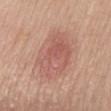Notes:
– workup — imaged on a skin check; not biopsied
– imaging modality — 15 mm crop, total-body photography
– lesion size — about 7 mm
– automated metrics — a lesion area of about 28 mm² and an outline eccentricity of about 0.7 (0 = round, 1 = elongated)
– patient — female, aged around 60
– site — the mid back